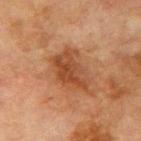Case summary:
– workup — no biopsy performed (imaged during a skin exam)
– acquisition — ~15 mm crop, total-body skin-cancer survey
– site — the right upper arm
– patient — male, in their mid-70s
– lesion size — about 5.5 mm